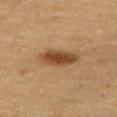Part of a total-body skin-imaging series; this lesion was reviewed on a skin check and was not flagged for biopsy. A female patient, roughly 55 years of age. Captured under cross-polarized illumination. The lesion's longest dimension is about 5 mm. A 15 mm close-up tile from a total-body photography series done for melanoma screening. The lesion is located on the right thigh.